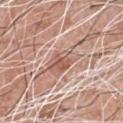Q: Is there a histopathology result?
A: imaged on a skin check; not biopsied
Q: What did automated image analysis measure?
A: border irregularity of about 3.5 on a 0–10 scale, a within-lesion color-variation index near 4.5/10, and a peripheral color-asymmetry measure near 1.5
Q: Lesion location?
A: the chest
Q: Illumination type?
A: white-light
Q: What are the patient's age and sex?
A: male, roughly 60 years of age
Q: What kind of image is this?
A: total-body-photography crop, ~15 mm field of view
Q: How large is the lesion?
A: ≈4 mm